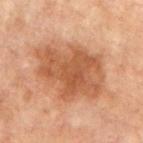This lesion was catalogued during total-body skin photography and was not selected for biopsy.